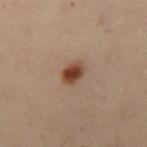Part of a total-body skin-imaging series; this lesion was reviewed on a skin check and was not flagged for biopsy.
A roughly 15 mm field-of-view crop from a total-body skin photograph.
A female patient, in their 30s.
Automated tile analysis of the lesion measured a mean CIELAB color near L≈34 a*≈17 b*≈25 and a lesion-to-skin contrast of about 11 (normalized; higher = more distinct). The analysis additionally found a nevus-likeness score of about 100/100.
This is a cross-polarized tile.
The lesion is located on the leg.